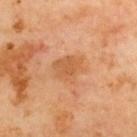Recorded during total-body skin imaging; not selected for excision or biopsy. Approximately 4 mm at its widest. Located on the upper back. The patient is a male in their 70s. Automated image analysis of the tile measured a lesion area of about 9 mm² and a symmetry-axis asymmetry near 0.25. It also reported an automated nevus-likeness rating near 0 out of 100 and a lesion-detection confidence of about 100/100. This is a cross-polarized tile. Cropped from a total-body skin-imaging series; the visible field is about 15 mm.